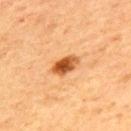Findings:
– workup: imaged on a skin check; not biopsied
– imaging modality: 15 mm crop, total-body photography
– subject: male, aged 63–67
– body site: the upper back
– lighting: cross-polarized
– automated lesion analysis: an average lesion color of about L≈49 a*≈26 b*≈40 (CIELAB) and about 15 CIELAB-L* units darker than the surrounding skin; a nevus-likeness score of about 100/100 and lesion-presence confidence of about 100/100
– size: about 3.5 mm